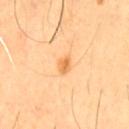Q: Was this lesion biopsied?
A: no biopsy performed (imaged during a skin exam)
Q: Patient demographics?
A: male, about 60 years old
Q: What is the anatomic site?
A: the chest
Q: What is the imaging modality?
A: total-body-photography crop, ~15 mm field of view
Q: What is the lesion's diameter?
A: ≈2 mm